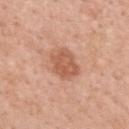Findings:
– biopsy status — no biopsy performed (imaged during a skin exam)
– patient — female, in their 50s
– acquisition — 15 mm crop, total-body photography
– automated metrics — a lesion area of about 9 mm², an outline eccentricity of about 0.6 (0 = round, 1 = elongated), and a symmetry-axis asymmetry near 0.2; an average lesion color of about L≈58 a*≈25 b*≈33 (CIELAB), a lesion–skin lightness drop of about 10, and a lesion-to-skin contrast of about 7 (normalized; higher = more distinct); a border-irregularity rating of about 2/10, a within-lesion color-variation index near 2.5/10, and a peripheral color-asymmetry measure near 1
– diameter — about 4 mm
– location — the upper back
– illumination — white-light